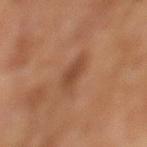biopsy_status: not biopsied; imaged during a skin examination
patient:
  sex: female
  age_approx: 60
image:
  source: total-body photography crop
  field_of_view_mm: 15
site: left lower leg
lighting: cross-polarized
automated_metrics:
  area_mm2_approx: 4.5
  shape_asymmetry: 0.3